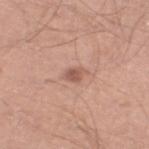biopsy status: total-body-photography surveillance lesion; no biopsy | patient: male, about 40 years old | tile lighting: white-light | anatomic site: the left lower leg | image source: ~15 mm crop, total-body skin-cancer survey | automated lesion analysis: a lesion area of about 3.5 mm², an outline eccentricity of about 0.8 (0 = round, 1 = elongated), and a symmetry-axis asymmetry near 0.25; a nevus-likeness score of about 10/100 and lesion-presence confidence of about 100/100.